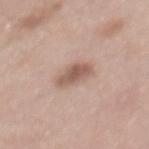Notes:
- biopsy status — catalogued during a skin exam; not biopsied
- imaging modality — ~15 mm tile from a whole-body skin photo
- site — the mid back
- patient — female, aged approximately 65